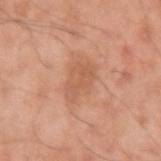The lesion was photographed on a routine skin check and not biopsied; there is no pathology result. On the left upper arm. Captured under white-light illumination. A lesion tile, about 15 mm wide, cut from a 3D total-body photograph. A male patient, aged around 55. Measured at roughly 4 mm in maximum diameter.